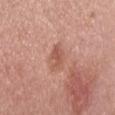Impression:
The lesion was photographed on a routine skin check and not biopsied; there is no pathology result.
Context:
Automated tile analysis of the lesion measured border irregularity of about 4 on a 0–10 scale. A male patient aged around 30. Imaged with white-light lighting. This image is a 15 mm lesion crop taken from a total-body photograph.